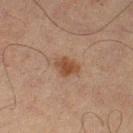Recorded during total-body skin imaging; not selected for excision or biopsy.
Approximately 3 mm at its widest.
Cropped from a whole-body photographic skin survey; the tile spans about 15 mm.
Captured under cross-polarized illumination.
Located on the left lower leg.
Automated tile analysis of the lesion measured an eccentricity of roughly 0.7. The analysis additionally found a mean CIELAB color near L≈38 a*≈17 b*≈26, roughly 8 lightness units darker than nearby skin, and a normalized lesion–skin contrast near 8. And it measured a border-irregularity index near 2.5/10 and peripheral color asymmetry of about 1.
The patient is a male approximately 65 years of age.